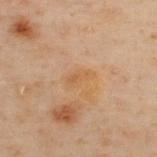  image:
    source: total-body photography crop
    field_of_view_mm: 15
  lesion_size:
    long_diameter_mm_approx: 3.0
  site: upper back
  lighting: cross-polarized
  patient:
    sex: male
    age_approx: 50